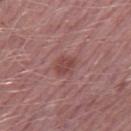Q: Patient demographics?
A: male, aged approximately 50
Q: Illumination type?
A: white-light
Q: Lesion size?
A: ~2.5 mm (longest diameter)
Q: How was this image acquired?
A: total-body-photography crop, ~15 mm field of view
Q: What is the anatomic site?
A: the leg
Q: What did automated image analysis measure?
A: an outline eccentricity of about 0.6 (0 = round, 1 = elongated) and a shape-asymmetry score of about 0.2 (0 = symmetric); about 8 CIELAB-L* units darker than the surrounding skin and a normalized border contrast of about 6.5; internal color variation of about 2.5 on a 0–10 scale and peripheral color asymmetry of about 1; an automated nevus-likeness rating near 25 out of 100 and lesion-presence confidence of about 100/100